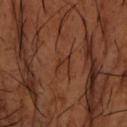No biopsy was performed on this lesion — it was imaged during a full skin examination and was not determined to be concerning.
A close-up tile cropped from a whole-body skin photograph, about 15 mm across.
On the left forearm.
Automated tile analysis of the lesion measured a shape eccentricity near 0.9 and a shape-asymmetry score of about 0.45 (0 = symmetric). The software also gave roughly 6 lightness units darker than nearby skin and a normalized border contrast of about 6. And it measured a border-irregularity index near 4.5/10 and a peripheral color-asymmetry measure near 0. And it measured a nevus-likeness score of about 0/100 and a detector confidence of about 65 out of 100 that the crop contains a lesion.
The patient is a male roughly 65 years of age.